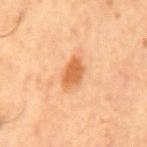<record>
  <biopsy_status>not biopsied; imaged during a skin examination</biopsy_status>
</record>